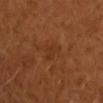Q: Was this lesion biopsied?
A: catalogued during a skin exam; not biopsied
Q: What is the anatomic site?
A: the arm
Q: Automated lesion metrics?
A: two-axis asymmetry of about 0.55; about 5 CIELAB-L* units darker than the surrounding skin and a lesion-to-skin contrast of about 4.5 (normalized; higher = more distinct); an automated nevus-likeness rating near 0 out of 100
Q: How was this image acquired?
A: 15 mm crop, total-body photography
Q: What lighting was used for the tile?
A: cross-polarized
Q: How large is the lesion?
A: about 3 mm
Q: Patient demographics?
A: female, aged 53 to 57